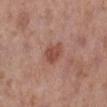{
  "biopsy_status": "not biopsied; imaged during a skin examination",
  "lighting": "white-light",
  "automated_metrics": {
    "color_variation_0_10": 2.5,
    "nevus_likeness_0_100": 65,
    "lesion_detection_confidence_0_100": 100
  },
  "patient": {
    "sex": "female",
    "age_approx": 40
  },
  "image": {
    "source": "total-body photography crop",
    "field_of_view_mm": 15
  },
  "site": "left lower leg"
}